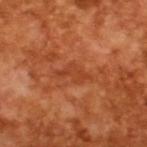Clinical impression: Captured during whole-body skin photography for melanoma surveillance; the lesion was not biopsied. Context: The tile uses cross-polarized illumination. About 4 mm across. A 15 mm close-up extracted from a 3D total-body photography capture. A male patient in their mid-60s.